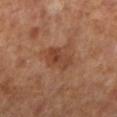Recorded during total-body skin imaging; not selected for excision or biopsy. The patient is a female aged 63 to 67. Longest diameter approximately 4 mm. Located on the left lower leg. A 15 mm crop from a total-body photograph taken for skin-cancer surveillance. This is a cross-polarized tile. The lesion-visualizer software estimated a lesion-to-skin contrast of about 6.5 (normalized; higher = more distinct).